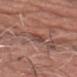Part of a total-body skin-imaging series; this lesion was reviewed on a skin check and was not flagged for biopsy.
From the chest.
The subject is a male aged around 65.
Cropped from a whole-body photographic skin survey; the tile spans about 15 mm.